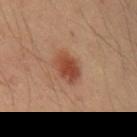Notes:
* notes: total-body-photography surveillance lesion; no biopsy
* image source: total-body-photography crop, ~15 mm field of view
* lesion size: ~3.5 mm (longest diameter)
* location: the arm
* subject: male, about 40 years old
* tile lighting: cross-polarized